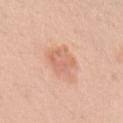Case summary:
– follow-up — no biopsy performed (imaged during a skin exam)
– automated metrics — an area of roughly 6.5 mm² and a symmetry-axis asymmetry near 0.2; a lesion–skin lightness drop of about 8 and a normalized lesion–skin contrast near 5
– image — ~15 mm crop, total-body skin-cancer survey
– body site — the left upper arm
– subject — female, roughly 25 years of age
– lesion diameter — ~3.5 mm (longest diameter)